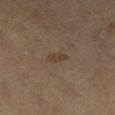<tbp_lesion>
  <biopsy_status>not biopsied; imaged during a skin examination</biopsy_status>
  <patient>
    <age_approx>60</age_approx>
  </patient>
  <image>
    <source>total-body photography crop</source>
    <field_of_view_mm>15</field_of_view_mm>
  </image>
  <lighting>cross-polarized</lighting>
  <lesion_size>
    <long_diameter_mm_approx>2.5</long_diameter_mm_approx>
  </lesion_size>
  <site>right lower leg</site>
</tbp_lesion>